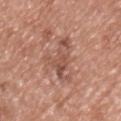Captured during whole-body skin photography for melanoma surveillance; the lesion was not biopsied. Cropped from a whole-body photographic skin survey; the tile spans about 15 mm. This is a white-light tile. On the chest. The lesion's longest dimension is about 5.5 mm. The patient is a male aged around 50.